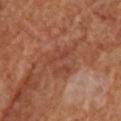Captured during whole-body skin photography for melanoma surveillance; the lesion was not biopsied.
An algorithmic analysis of the crop reported a lesion area of about 8.5 mm². The analysis additionally found an automated nevus-likeness rating near 0 out of 100 and a lesion-detection confidence of about 95/100.
The lesion is on the upper back.
The patient is a female approximately 65 years of age.
This is a cross-polarized tile.
Longest diameter approximately 4.5 mm.
A close-up tile cropped from a whole-body skin photograph, about 15 mm across.